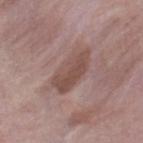workup: imaged on a skin check; not biopsied
diameter: ≈5.5 mm
subject: male, about 40 years old
imaging modality: 15 mm crop, total-body photography
lighting: white-light
image-analysis metrics: a border-irregularity rating of about 3/10, a within-lesion color-variation index near 3/10, and a peripheral color-asymmetry measure near 1; an automated nevus-likeness rating near 10 out of 100 and a detector confidence of about 100 out of 100 that the crop contains a lesion
anatomic site: the right lower leg